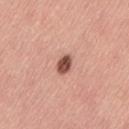Q: Was a biopsy performed?
A: catalogued during a skin exam; not biopsied
Q: What is the lesion's diameter?
A: about 3 mm
Q: How was the tile lit?
A: white-light illumination
Q: What kind of image is this?
A: ~15 mm crop, total-body skin-cancer survey
Q: What did automated image analysis measure?
A: an average lesion color of about L≈51 a*≈25 b*≈27 (CIELAB); a border-irregularity rating of about 1.5/10 and a within-lesion color-variation index near 3.5/10; a classifier nevus-likeness of about 90/100 and a lesion-detection confidence of about 100/100
Q: Lesion location?
A: the left thigh
Q: Who is the patient?
A: female, in their mid- to late 40s